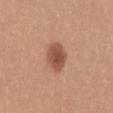Part of a total-body skin-imaging series; this lesion was reviewed on a skin check and was not flagged for biopsy. The subject is a female about 30 years old. The lesion is on the mid back. A region of skin cropped from a whole-body photographic capture, roughly 15 mm wide.